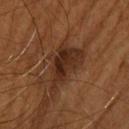Q: What did automated image analysis measure?
A: an area of roughly 15 mm², an outline eccentricity of about 0.85 (0 = round, 1 = elongated), and a shape-asymmetry score of about 0.55 (0 = symmetric)
Q: Patient demographics?
A: male, aged approximately 60
Q: What kind of image is this?
A: 15 mm crop, total-body photography
Q: Illumination type?
A: cross-polarized
Q: What is the anatomic site?
A: the upper back
Q: Lesion size?
A: about 6.5 mm